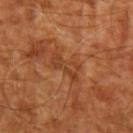biopsy_status: not biopsied; imaged during a skin examination
lesion_size:
  long_diameter_mm_approx: 4.0
site: left upper arm
image:
  source: total-body photography crop
  field_of_view_mm: 15
patient:
  age_approx: 65